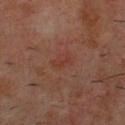The lesion was photographed on a routine skin check and not biopsied; there is no pathology result. The lesion is on the chest. This image is a 15 mm lesion crop taken from a total-body photograph. A male subject, aged approximately 60.